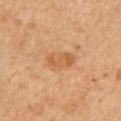Q: Was a biopsy performed?
A: total-body-photography surveillance lesion; no biopsy
Q: What is the imaging modality?
A: ~15 mm crop, total-body skin-cancer survey
Q: What is the anatomic site?
A: the chest
Q: Who is the patient?
A: male, aged approximately 60